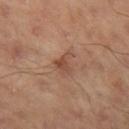The lesion was photographed on a routine skin check and not biopsied; there is no pathology result. The lesion is on the left thigh. About 3 mm across. A 15 mm crop from a total-body photograph taken for skin-cancer surveillance.